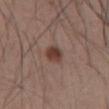  biopsy_status: not biopsied; imaged during a skin examination
  lighting: white-light
  automated_metrics:
    area_mm2_approx: 5.0
    eccentricity: 0.45
    shape_asymmetry: 0.2
    vs_skin_darker_L: 11.0
    vs_skin_contrast_norm: 9.0
    border_irregularity_0_10: 1.5
    color_variation_0_10: 4.0
  image:
    source: total-body photography crop
    field_of_view_mm: 15
  patient:
    sex: male
    age_approx: 55
  lesion_size:
    long_diameter_mm_approx: 2.5
  site: abdomen